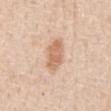• follow-up: total-body-photography surveillance lesion; no biopsy
• diameter: ~4 mm (longest diameter)
• illumination: white-light illumination
• acquisition: 15 mm crop, total-body photography
• TBP lesion metrics: a symmetry-axis asymmetry near 0.2
• patient: male, aged around 60
• site: the abdomen